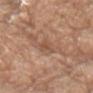{
  "biopsy_status": "not biopsied; imaged during a skin examination",
  "lighting": "white-light",
  "site": "head or neck",
  "patient": {
    "sex": "male",
    "age_approx": "85+"
  },
  "image": {
    "source": "total-body photography crop",
    "field_of_view_mm": 15
  }
}